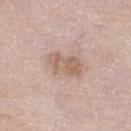Q: Was this lesion biopsied?
A: total-body-photography surveillance lesion; no biopsy
Q: What is the anatomic site?
A: the right lower leg
Q: What lighting was used for the tile?
A: white-light
Q: What is the imaging modality?
A: 15 mm crop, total-body photography
Q: What are the patient's age and sex?
A: male, aged approximately 80
Q: Lesion size?
A: ≈4 mm